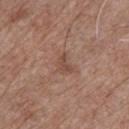The lesion was photographed on a routine skin check and not biopsied; there is no pathology result. The lesion-visualizer software estimated a lesion area of about 3.5 mm². It also reported a border-irregularity index near 4/10, a color-variation rating of about 1/10, and a peripheral color-asymmetry measure near 0.5. It also reported a classifier nevus-likeness of about 0/100 and lesion-presence confidence of about 100/100. A 15 mm close-up tile from a total-body photography series done for melanoma screening. A male subject, about 55 years old. Approximately 2.5 mm at its widest. The lesion is located on the chest.